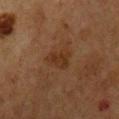This lesion was catalogued during total-body skin photography and was not selected for biopsy. The lesion's longest dimension is about 3.5 mm. A roughly 15 mm field-of-view crop from a total-body skin photograph. Captured under cross-polarized illumination. Located on the chest. A male patient about 75 years old.